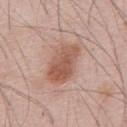Assessment: Recorded during total-body skin imaging; not selected for excision or biopsy. Context: The recorded lesion diameter is about 5.5 mm. The tile uses white-light illumination. Located on the front of the torso. A 15 mm close-up tile from a total-body photography series done for melanoma screening. An algorithmic analysis of the crop reported a within-lesion color-variation index near 4/10 and peripheral color asymmetry of about 1.5. A male patient in their mid- to late 50s.